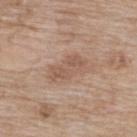Imaged during a routine full-body skin examination; the lesion was not biopsied and no histopathology is available. A close-up tile cropped from a whole-body skin photograph, about 15 mm across. A female subject in their mid- to late 70s. This is a white-light tile. Automated tile analysis of the lesion measured a mean CIELAB color near L≈55 a*≈18 b*≈28, a lesion–skin lightness drop of about 8, and a normalized lesion–skin contrast near 5.5. The analysis additionally found a within-lesion color-variation index near 2.5/10 and radial color variation of about 1. Measured at roughly 4.5 mm in maximum diameter. The lesion is located on the upper back.